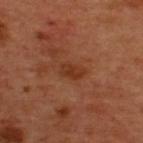<case>
<biopsy_status>not biopsied; imaged during a skin examination</biopsy_status>
<site>upper back</site>
<patient>
  <sex>male</sex>
  <age_approx>50</age_approx>
</patient>
<image>
  <source>total-body photography crop</source>
  <field_of_view_mm>15</field_of_view_mm>
</image>
</case>